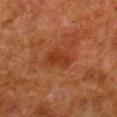{
  "biopsy_status": "not biopsied; imaged during a skin examination",
  "image": {
    "source": "total-body photography crop",
    "field_of_view_mm": 15
  },
  "site": "right lower leg",
  "lighting": "cross-polarized",
  "patient": {
    "sex": "male",
    "age_approx": 80
  }
}